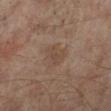The lesion was tiled from a total-body skin photograph and was not biopsied. The lesion is on the leg. An algorithmic analysis of the crop reported a border-irregularity index near 3.5/10, a color-variation rating of about 2/10, and radial color variation of about 0.5. The analysis additionally found an automated nevus-likeness rating near 0 out of 100. Measured at roughly 3.5 mm in maximum diameter. Imaged with cross-polarized lighting. The patient is a male approximately 65 years of age. A lesion tile, about 15 mm wide, cut from a 3D total-body photograph.